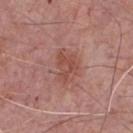Assessment: Captured during whole-body skin photography for melanoma surveillance; the lesion was not biopsied. Acquisition and patient details: A region of skin cropped from a whole-body photographic capture, roughly 15 mm wide. The lesion-visualizer software estimated two-axis asymmetry of about 0.35. From the front of the torso. The lesion's longest dimension is about 4 mm. A male patient aged 73 to 77. Captured under white-light illumination.